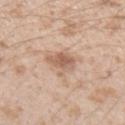From the arm.
A male patient aged approximately 25.
The tile uses white-light illumination.
A lesion tile, about 15 mm wide, cut from a 3D total-body photograph.
The lesion-visualizer software estimated an area of roughly 7 mm², a shape eccentricity near 0.5, and a shape-asymmetry score of about 0.3 (0 = symmetric). And it measured a within-lesion color-variation index near 4/10 and a peripheral color-asymmetry measure near 1. And it measured a nevus-likeness score of about 5/100 and lesion-presence confidence of about 100/100.